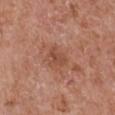Impression:
Recorded during total-body skin imaging; not selected for excision or biopsy.
Image and clinical context:
The patient is a male approximately 65 years of age. Imaged with white-light lighting. Cropped from a whole-body photographic skin survey; the tile spans about 15 mm. Located on the right upper arm.